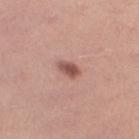<tbp_lesion>
  <biopsy_status>not biopsied; imaged during a skin examination</biopsy_status>
  <lighting>white-light</lighting>
  <automated_metrics>
    <cielab_L>52</cielab_L>
    <cielab_a>23</cielab_a>
    <cielab_b>25</cielab_b>
    <vs_skin_darker_L>13.0</vs_skin_darker_L>
    <vs_skin_contrast_norm>9.0</vs_skin_contrast_norm>
  </automated_metrics>
  <lesion_size>
    <long_diameter_mm_approx>2.5</long_diameter_mm_approx>
  </lesion_size>
  <image>
    <source>total-body photography crop</source>
    <field_of_view_mm>15</field_of_view_mm>
  </image>
  <site>left thigh</site>
  <patient>
    <sex>female</sex>
    <age_approx>25</age_approx>
  </patient>
</tbp_lesion>